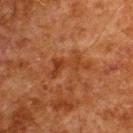Impression: This lesion was catalogued during total-body skin photography and was not selected for biopsy. Clinical summary: Imaged with cross-polarized lighting. A male subject, aged approximately 60. On the back. A close-up tile cropped from a whole-body skin photograph, about 15 mm across.